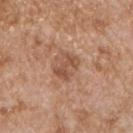<case>
  <biopsy_status>not biopsied; imaged during a skin examination</biopsy_status>
  <patient>
    <sex>male</sex>
    <age_approx>65</age_approx>
  </patient>
  <image>
    <source>total-body photography crop</source>
    <field_of_view_mm>15</field_of_view_mm>
  </image>
  <site>front of the torso</site>
</case>